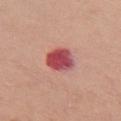Captured during whole-body skin photography for melanoma surveillance; the lesion was not biopsied. About 4 mm across. The tile uses white-light illumination. Cropped from a whole-body photographic skin survey; the tile spans about 15 mm. The patient is a female aged around 60. From the chest.